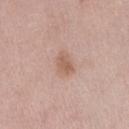<record>
<biopsy_status>not biopsied; imaged during a skin examination</biopsy_status>
<patient>
  <sex>female</sex>
  <age_approx>40</age_approx>
</patient>
<site>right thigh</site>
<lighting>white-light</lighting>
<image>
  <source>total-body photography crop</source>
  <field_of_view_mm>15</field_of_view_mm>
</image>
</record>